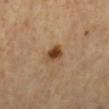site: left lower leg
patient:
  sex: female
  age_approx: 70
automated_metrics:
  cielab_L: 45
  cielab_a: 20
  cielab_b: 34
  vs_skin_contrast_norm: 10.5
  nevus_likeness_0_100: 95
lighting: cross-polarized
image:
  source: total-body photography crop
  field_of_view_mm: 15
lesion_size:
  long_diameter_mm_approx: 3.0